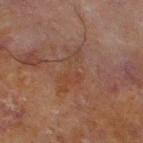Captured during whole-body skin photography for melanoma surveillance; the lesion was not biopsied. From the left lower leg. The lesion's longest dimension is about 5.5 mm. The subject is a male aged 68 to 72. The tile uses cross-polarized illumination. A close-up tile cropped from a whole-body skin photograph, about 15 mm across.